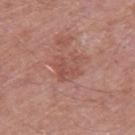Impression: Captured during whole-body skin photography for melanoma surveillance; the lesion was not biopsied. Background: A male patient, aged 78 to 82. A 15 mm crop from a total-body photograph taken for skin-cancer surveillance. The lesion is located on the right thigh.